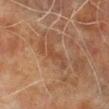No biopsy was performed on this lesion — it was imaged during a full skin examination and was not determined to be concerning.
The lesion is on the left lower leg.
The recorded lesion diameter is about 3 mm.
Cropped from a total-body skin-imaging series; the visible field is about 15 mm.
Captured under cross-polarized illumination.
The subject is a male aged approximately 70.